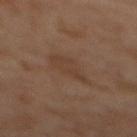  biopsy_status: not biopsied; imaged during a skin examination
  image:
    source: total-body photography crop
    field_of_view_mm: 15
  lesion_size:
    long_diameter_mm_approx: 5.0
  automated_metrics:
    border_irregularity_0_10: 3.5
    color_variation_0_10: 1.5
    peripheral_color_asymmetry: 0.5
    nevus_likeness_0_100: 0
    lesion_detection_confidence_0_100: 100
  patient:
    sex: female
    age_approx: 60
  site: back